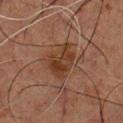Recorded during total-body skin imaging; not selected for excision or biopsy. The subject is a male approximately 50 years of age. The lesion-visualizer software estimated a lesion area of about 11 mm², an outline eccentricity of about 0.7 (0 = round, 1 = elongated), and two-axis asymmetry of about 0.35. And it measured an average lesion color of about L≈29 a*≈17 b*≈25 (CIELAB). It also reported a border-irregularity index near 4/10, internal color variation of about 4 on a 0–10 scale, and radial color variation of about 1.5. The software also gave lesion-presence confidence of about 100/100. A region of skin cropped from a whole-body photographic capture, roughly 15 mm wide. The lesion is located on the chest.